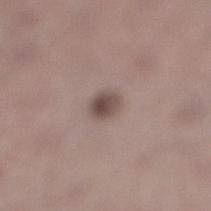{"image": {"source": "total-body photography crop", "field_of_view_mm": 15}, "site": "right lower leg", "lighting": "white-light", "lesion_size": {"long_diameter_mm_approx": 2.5}, "automated_metrics": {"cielab_L": 48, "cielab_a": 15, "cielab_b": 19, "vs_skin_darker_L": 12.0, "nevus_likeness_0_100": 85, "lesion_detection_confidence_0_100": 100}, "patient": {"sex": "male", "age_approx": 45}}